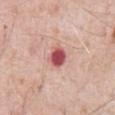Clinical impression: Part of a total-body skin-imaging series; this lesion was reviewed on a skin check and was not flagged for biopsy. Image and clinical context: From the abdomen. Imaged with white-light lighting. A 15 mm close-up tile from a total-body photography series done for melanoma screening. The lesion-visualizer software estimated a lesion color around L≈54 a*≈33 b*≈23 in CIELAB, roughly 17 lightness units darker than nearby skin, and a normalized lesion–skin contrast near 11. The software also gave a color-variation rating of about 3.5/10 and peripheral color asymmetry of about 1. The analysis additionally found an automated nevus-likeness rating near 0 out of 100. A male subject in their 80s. Measured at roughly 2.5 mm in maximum diameter.